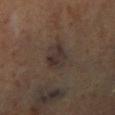notes: no biopsy performed (imaged during a skin exam) | illumination: cross-polarized illumination | patient: female, aged around 50 | lesion size: about 4.5 mm | body site: the left lower leg | acquisition: ~15 mm tile from a whole-body skin photo.